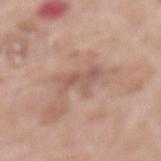{"biopsy_status": "not biopsied; imaged during a skin examination", "image": {"source": "total-body photography crop", "field_of_view_mm": 15}, "site": "mid back", "lighting": "white-light", "patient": {"sex": "female", "age_approx": 75}}